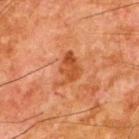Acquisition and patient details: The lesion is located on the upper back. The lesion-visualizer software estimated lesion-presence confidence of about 100/100. The lesion's longest dimension is about 4 mm. A male patient, aged approximately 65. A region of skin cropped from a whole-body photographic capture, roughly 15 mm wide.